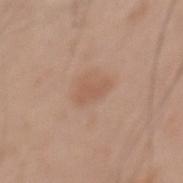Q: Is there a histopathology result?
A: no biopsy performed (imaged during a skin exam)
Q: What kind of image is this?
A: ~15 mm crop, total-body skin-cancer survey
Q: Lesion size?
A: ≈2.5 mm
Q: What did automated image analysis measure?
A: a footprint of about 3 mm², an outline eccentricity of about 0.85 (0 = round, 1 = elongated), and a shape-asymmetry score of about 0.35 (0 = symmetric); a lesion color around L≈56 a*≈20 b*≈30 in CIELAB
Q: Patient demographics?
A: male, aged approximately 65
Q: Lesion location?
A: the mid back